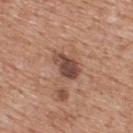Recorded during total-body skin imaging; not selected for excision or biopsy. The tile uses white-light illumination. The subject is a male aged 58–62. Automated image analysis of the tile measured an area of roughly 7 mm², an eccentricity of roughly 0.8, and a shape-asymmetry score of about 0.25 (0 = symmetric). And it measured a border-irregularity rating of about 2.5/10, a within-lesion color-variation index near 4.5/10, and peripheral color asymmetry of about 1.5. A roughly 15 mm field-of-view crop from a total-body skin photograph. The lesion is located on the back.